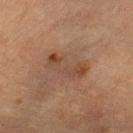{
  "biopsy_status": "not biopsied; imaged during a skin examination",
  "patient": {
    "sex": "male",
    "age_approx": "85+"
  },
  "automated_metrics": {
    "area_mm2_approx": 10.0,
    "eccentricity": 0.85
  },
  "site": "leg",
  "lighting": "cross-polarized",
  "image": {
    "source": "total-body photography crop",
    "field_of_view_mm": 15
  },
  "lesion_size": {
    "long_diameter_mm_approx": 4.5
  }
}